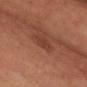Findings:
– biopsy status: no biopsy performed (imaged during a skin exam)
– subject: male, aged approximately 65
– automated metrics: an area of roughly 3.5 mm², a shape eccentricity near 0.65, and a symmetry-axis asymmetry near 0.25; an automated nevus-likeness rating near 0 out of 100 and a lesion-detection confidence of about 100/100
– illumination: cross-polarized
– location: the chest
– image: total-body-photography crop, ~15 mm field of view